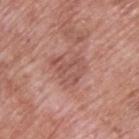This lesion was catalogued during total-body skin photography and was not selected for biopsy.
A 15 mm close-up extracted from a 3D total-body photography capture.
Captured under white-light illumination.
Located on the upper back.
Longest diameter approximately 4 mm.
The subject is a male approximately 70 years of age.